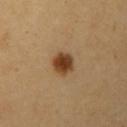The lesion was tiled from a total-body skin photograph and was not biopsied. The total-body-photography lesion software estimated a lesion area of about 6.5 mm², an eccentricity of roughly 0.35, and two-axis asymmetry of about 0.2. And it measured a mean CIELAB color near L≈43 a*≈20 b*≈36 and a lesion-to-skin contrast of about 11.5 (normalized; higher = more distinct). The software also gave border irregularity of about 1.5 on a 0–10 scale and a color-variation rating of about 4.5/10. The software also gave an automated nevus-likeness rating near 100 out of 100. Imaged with cross-polarized lighting. A 15 mm close-up tile from a total-body photography series done for melanoma screening. The lesion is on the left upper arm. A female subject, roughly 60 years of age.